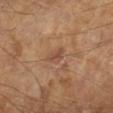| feature | finding |
|---|---|
| notes | catalogued during a skin exam; not biopsied |
| acquisition | 15 mm crop, total-body photography |
| subject | male, aged 63–67 |
| image-analysis metrics | a border-irregularity rating of about 3.5/10 and a peripheral color-asymmetry measure near 0.5; an automated nevus-likeness rating near 5 out of 100 and lesion-presence confidence of about 100/100 |
| diameter | ≈2.5 mm |